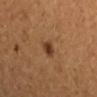This lesion was catalogued during total-body skin photography and was not selected for biopsy. Located on the arm. A female subject, about 35 years old. The tile uses cross-polarized illumination. Cropped from a whole-body photographic skin survey; the tile spans about 15 mm. Approximately 2 mm at its widest.